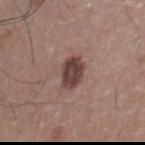Part of a total-body skin-imaging series; this lesion was reviewed on a skin check and was not flagged for biopsy. Approximately 3.5 mm at its widest. Cropped from a total-body skin-imaging series; the visible field is about 15 mm. On the front of the torso. A male subject about 40 years old. This is a white-light tile. The total-body-photography lesion software estimated a shape eccentricity near 0.75 and a shape-asymmetry score of about 0.2 (0 = symmetric). It also reported an average lesion color of about L≈42 a*≈18 b*≈21 (CIELAB), roughly 14 lightness units darker than nearby skin, and a lesion-to-skin contrast of about 10.5 (normalized; higher = more distinct). And it measured a border-irregularity index near 2/10, internal color variation of about 3 on a 0–10 scale, and a peripheral color-asymmetry measure near 1.